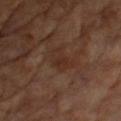| field | value |
|---|---|
| follow-up | total-body-photography surveillance lesion; no biopsy |
| location | the front of the torso |
| patient | male, aged approximately 65 |
| size | ≈3.5 mm |
| tile lighting | cross-polarized |
| image | 15 mm crop, total-body photography |